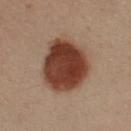Imaged during a routine full-body skin examination; the lesion was not biopsied and no histopathology is available. A male patient, aged approximately 50. Captured under cross-polarized illumination. On the left forearm. A 15 mm crop from a total-body photograph taken for skin-cancer surveillance.